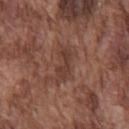biopsy status: total-body-photography surveillance lesion; no biopsy | body site: the chest | patient: male, aged 73–77 | lighting: white-light | image: total-body-photography crop, ~15 mm field of view | lesion size: ~4.5 mm (longest diameter).